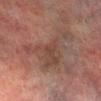Q: Was a biopsy performed?
A: no biopsy performed (imaged during a skin exam)
Q: What kind of image is this?
A: ~15 mm crop, total-body skin-cancer survey
Q: What are the patient's age and sex?
A: male, approximately 75 years of age
Q: Lesion location?
A: the right lower leg
Q: How was the tile lit?
A: cross-polarized illumination
Q: What did automated image analysis measure?
A: a lesion area of about 18 mm², an eccentricity of roughly 0.8, and a shape-asymmetry score of about 0.7 (0 = symmetric); a border-irregularity rating of about 9.5/10 and peripheral color asymmetry of about 1; a classifier nevus-likeness of about 0/100